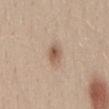No biopsy was performed on this lesion — it was imaged during a full skin examination and was not determined to be concerning. The patient is a female in their 30s. A close-up tile cropped from a whole-body skin photograph, about 15 mm across. The lesion is on the mid back. Automated tile analysis of the lesion measured a footprint of about 4.5 mm², an outline eccentricity of about 0.6 (0 = round, 1 = elongated), and two-axis asymmetry of about 0.25. Captured under white-light illumination. Approximately 2.5 mm at its widest.